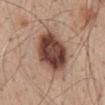follow-up — catalogued during a skin exam; not biopsied | subject — male, aged approximately 50 | anatomic site — the mid back | imaging modality — total-body-photography crop, ~15 mm field of view.